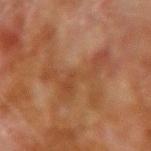Recorded during total-body skin imaging; not selected for excision or biopsy. A region of skin cropped from a whole-body photographic capture, roughly 15 mm wide. On the right upper arm. A male patient in their 70s.